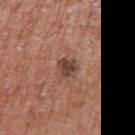biopsy status: catalogued during a skin exam; not biopsied | anatomic site: the right upper arm | patient: male, about 75 years old | lesion diameter: ≈2.5 mm | image source: ~15 mm crop, total-body skin-cancer survey | tile lighting: white-light.